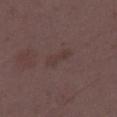{
  "biopsy_status": "not biopsied; imaged during a skin examination",
  "lesion_size": {
    "long_diameter_mm_approx": 4.0
  },
  "site": "leg",
  "automated_metrics": {
    "cielab_L": 36,
    "cielab_a": 15,
    "cielab_b": 17,
    "vs_skin_contrast_norm": 4.5,
    "border_irregularity_0_10": 3.5,
    "color_variation_0_10": 2.0,
    "peripheral_color_asymmetry": 0.5,
    "nevus_likeness_0_100": 0,
    "lesion_detection_confidence_0_100": 100
  },
  "image": {
    "source": "total-body photography crop",
    "field_of_view_mm": 15
  },
  "patient": {
    "sex": "female",
    "age_approx": 35
  },
  "lighting": "white-light"
}